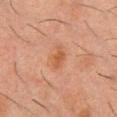Imaged during a routine full-body skin examination; the lesion was not biopsied and no histopathology is available. The lesion is on the chest. A close-up tile cropped from a whole-body skin photograph, about 15 mm across. The subject is a male aged around 50. Longest diameter approximately 3 mm. The tile uses cross-polarized illumination.